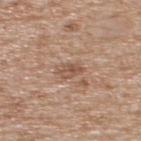Q: Was a biopsy performed?
A: imaged on a skin check; not biopsied
Q: What is the anatomic site?
A: the upper back
Q: How was this image acquired?
A: ~15 mm crop, total-body skin-cancer survey
Q: What did automated image analysis measure?
A: an automated nevus-likeness rating near 0 out of 100
Q: Patient demographics?
A: male, in their mid- to late 60s
Q: What is the lesion's diameter?
A: ~2.5 mm (longest diameter)